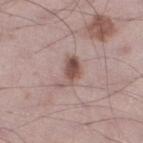No biopsy was performed on this lesion — it was imaged during a full skin examination and was not determined to be concerning.
The lesion is on the left lower leg.
A 15 mm crop from a total-body photograph taken for skin-cancer surveillance.
The total-body-photography lesion software estimated a footprint of about 5.5 mm², an eccentricity of roughly 0.85, and two-axis asymmetry of about 0.45. The analysis additionally found a border-irregularity rating of about 5.5/10, a color-variation rating of about 3/10, and a peripheral color-asymmetry measure near 1. The analysis additionally found an automated nevus-likeness rating near 85 out of 100 and a detector confidence of about 100 out of 100 that the crop contains a lesion.
A male subject roughly 70 years of age.
The tile uses white-light illumination.
The lesion's longest dimension is about 4 mm.